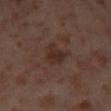Recorded during total-body skin imaging; not selected for excision or biopsy.
On the left thigh.
A female patient, aged 53–57.
A close-up tile cropped from a whole-body skin photograph, about 15 mm across.
Measured at roughly 3 mm in maximum diameter.